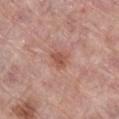Assessment: Recorded during total-body skin imaging; not selected for excision or biopsy. Context: Measured at roughly 2.5 mm in maximum diameter. The lesion-visualizer software estimated a classifier nevus-likeness of about 50/100 and a detector confidence of about 100 out of 100 that the crop contains a lesion. The tile uses white-light illumination. The lesion is located on the leg. A female patient, about 60 years old. A lesion tile, about 15 mm wide, cut from a 3D total-body photograph.